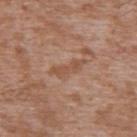Acquisition and patient details:
The lesion is located on the upper back. Measured at roughly 4 mm in maximum diameter. A male subject, roughly 45 years of age. A 15 mm close-up extracted from a 3D total-body photography capture.